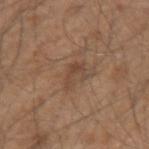biopsy status=no biopsy performed (imaged during a skin exam) | anatomic site=the left upper arm | patient=male, in their 50s | diameter=~3 mm (longest diameter) | illumination=white-light | image source=~15 mm crop, total-body skin-cancer survey.